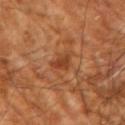<case>
<biopsy_status>not biopsied; imaged during a skin examination</biopsy_status>
<site>left forearm</site>
<patient>
  <age_approx>65</age_approx>
</patient>
<image>
  <source>total-body photography crop</source>
  <field_of_view_mm>15</field_of_view_mm>
</image>
<lesion_size>
  <long_diameter_mm_approx>3.0</long_diameter_mm_approx>
</lesion_size>
</case>